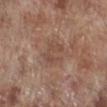Part of a total-body skin-imaging series; this lesion was reviewed on a skin check and was not flagged for biopsy. A region of skin cropped from a whole-body photographic capture, roughly 15 mm wide. On the leg. A male subject, roughly 70 years of age.